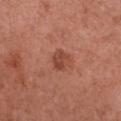Part of a total-body skin-imaging series; this lesion was reviewed on a skin check and was not flagged for biopsy. Located on the chest. The subject is a female aged 48–52. This image is a 15 mm lesion crop taken from a total-body photograph.